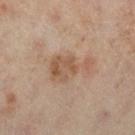Acquisition and patient details:
A female subject, aged approximately 55. The lesion-visualizer software estimated an area of roughly 11 mm² and an outline eccentricity of about 0.85 (0 = round, 1 = elongated). The software also gave a lesion color around L≈45 a*≈15 b*≈26 in CIELAB and a lesion–skin lightness drop of about 7. And it measured a within-lesion color-variation index near 3.5/10 and radial color variation of about 1. The analysis additionally found a lesion-detection confidence of about 100/100. This is a cross-polarized tile. A region of skin cropped from a whole-body photographic capture, roughly 15 mm wide. Located on the left leg.